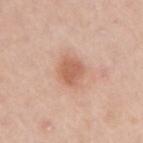Q: Was a biopsy performed?
A: total-body-photography surveillance lesion; no biopsy
Q: Automated lesion metrics?
A: an outline eccentricity of about 0.65 (0 = round, 1 = elongated); an average lesion color of about L≈61 a*≈24 b*≈32 (CIELAB) and a normalized border contrast of about 7; an automated nevus-likeness rating near 80 out of 100 and lesion-presence confidence of about 100/100
Q: Who is the patient?
A: female, aged approximately 45
Q: How was the tile lit?
A: white-light
Q: Where on the body is the lesion?
A: the right upper arm
Q: What is the lesion's diameter?
A: ~3.5 mm (longest diameter)
Q: What is the imaging modality?
A: 15 mm crop, total-body photography